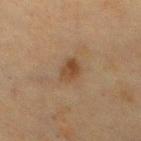notes: catalogued during a skin exam; not biopsied | acquisition: ~15 mm crop, total-body skin-cancer survey | location: the chest | diameter: about 3.5 mm | subject: female, aged around 55 | automated metrics: an eccentricity of roughly 0.8 and two-axis asymmetry of about 0.3; roughly 7 lightness units darker than nearby skin and a normalized lesion–skin contrast near 7.